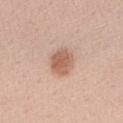Clinical summary: Automated tile analysis of the lesion measured a lesion color around L≈60 a*≈21 b*≈29 in CIELAB, about 12 CIELAB-L* units darker than the surrounding skin, and a normalized border contrast of about 7.5. The software also gave border irregularity of about 2 on a 0–10 scale and peripheral color asymmetry of about 0.5. The software also gave a classifier nevus-likeness of about 95/100 and lesion-presence confidence of about 100/100. The recorded lesion diameter is about 4 mm. Cropped from a total-body skin-imaging series; the visible field is about 15 mm. The patient is a female aged around 40. Captured under white-light illumination. On the right forearm.